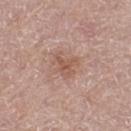This lesion was catalogued during total-body skin photography and was not selected for biopsy. Located on the left thigh. The subject is a female in their mid- to late 70s. A 15 mm close-up tile from a total-body photography series done for melanoma screening.